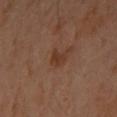<tbp_lesion>
<biopsy_status>not biopsied; imaged during a skin examination</biopsy_status>
<lesion_size>
  <long_diameter_mm_approx>3.0</long_diameter_mm_approx>
</lesion_size>
<image>
  <source>total-body photography crop</source>
  <field_of_view_mm>15</field_of_view_mm>
</image>
<site>front of the torso</site>
<lighting>cross-polarized</lighting>
<patient>
  <sex>female</sex>
  <age_approx>60</age_approx>
</patient>
<automated_metrics>
  <color_variation_0_10>2.5</color_variation_0_10>
  <peripheral_color_asymmetry>1.0</peripheral_color_asymmetry>
</automated_metrics>
</tbp_lesion>